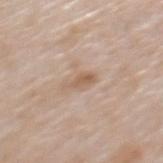size: ≈3 mm
body site: the mid back
tile lighting: white-light illumination
automated lesion analysis: a lesion color around L≈59 a*≈16 b*≈30 in CIELAB, a lesion–skin lightness drop of about 8, and a lesion-to-skin contrast of about 6 (normalized; higher = more distinct)
subject: male, aged 63–67
imaging modality: total-body-photography crop, ~15 mm field of view